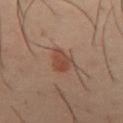The lesion was tiled from a total-body skin photograph and was not biopsied.
An algorithmic analysis of the crop reported a mean CIELAB color near L≈44 a*≈20 b*≈28, about 8 CIELAB-L* units darker than the surrounding skin, and a normalized lesion–skin contrast near 7.
Approximately 3.5 mm at its widest.
The subject is a male in their 40s.
The lesion is on the chest.
A lesion tile, about 15 mm wide, cut from a 3D total-body photograph.
Captured under cross-polarized illumination.